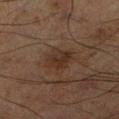Notes:
• follow-up — total-body-photography surveillance lesion; no biopsy
• subject — male, approximately 70 years of age
• image source — ~15 mm crop, total-body skin-cancer survey
• lighting — cross-polarized illumination
• size — ≈3 mm
• location — the right lower leg